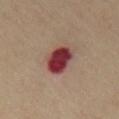Clinical impression: This lesion was catalogued during total-body skin photography and was not selected for biopsy. Context: The subject is a male roughly 55 years of age. Approximately 4.5 mm at its widest. Located on the chest. This image is a 15 mm lesion crop taken from a total-body photograph.